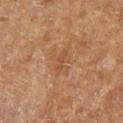Part of a total-body skin-imaging series; this lesion was reviewed on a skin check and was not flagged for biopsy. The lesion is on the right forearm. The lesion's longest dimension is about 3 mm. A roughly 15 mm field-of-view crop from a total-body skin photograph. Captured under cross-polarized illumination. A female subject, aged around 70.